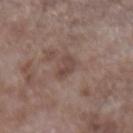notes = total-body-photography surveillance lesion; no biopsy | site = the left forearm | tile lighting = white-light illumination | size = about 3 mm | patient = male, in their mid- to late 60s | imaging modality = 15 mm crop, total-body photography.